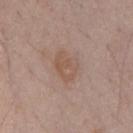Acquisition and patient details:
Cropped from a whole-body photographic skin survey; the tile spans about 15 mm. An algorithmic analysis of the crop reported an area of roughly 8 mm², an eccentricity of roughly 0.7, and two-axis asymmetry of about 0.25. The analysis additionally found a mean CIELAB color near L≈54 a*≈17 b*≈26, a lesion–skin lightness drop of about 6, and a normalized border contrast of about 5.5. The analysis additionally found a border-irregularity index near 2.5/10, internal color variation of about 2.5 on a 0–10 scale, and radial color variation of about 1. It also reported a nevus-likeness score of about 0/100. Approximately 3.5 mm at its widest. A male patient, aged 63–67. Located on the chest.